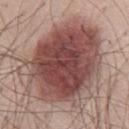follow-up: imaged on a skin check; not biopsied | site: the chest | lesion size: ≈11 mm | patient: male, aged 68–72 | imaging modality: 15 mm crop, total-body photography.